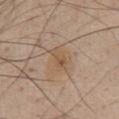Assessment:
The lesion was photographed on a routine skin check and not biopsied; there is no pathology result.
Acquisition and patient details:
Imaged with white-light lighting. A male subject, aged 53 to 57. The total-body-photography lesion software estimated a lesion area of about 4.5 mm², a shape eccentricity near 0.85, and two-axis asymmetry of about 0.35. The software also gave an average lesion color of about L≈54 a*≈16 b*≈32 (CIELAB), a lesion–skin lightness drop of about 7, and a normalized lesion–skin contrast near 6. It also reported a border-irregularity rating of about 4/10, a color-variation rating of about 4/10, and a peripheral color-asymmetry measure near 1.5. A 15 mm close-up extracted from a 3D total-body photography capture. The lesion is on the front of the torso.